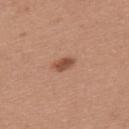This lesion was catalogued during total-body skin photography and was not selected for biopsy. Automated image analysis of the tile measured an area of roughly 3.5 mm² and an outline eccentricity of about 0.85 (0 = round, 1 = elongated). The software also gave a lesion color around L≈50 a*≈23 b*≈31 in CIELAB, roughly 12 lightness units darker than nearby skin, and a lesion-to-skin contrast of about 8.5 (normalized; higher = more distinct). A close-up tile cropped from a whole-body skin photograph, about 15 mm across. On the back. Captured under white-light illumination. The lesion's longest dimension is about 3 mm. A female subject, aged approximately 35.